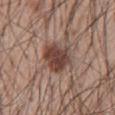<case>
<biopsy_status>not biopsied; imaged during a skin examination</biopsy_status>
<lighting>white-light</lighting>
<image>
  <source>total-body photography crop</source>
  <field_of_view_mm>15</field_of_view_mm>
</image>
<site>chest</site>
<patient>
  <sex>male</sex>
  <age_approx>55</age_approx>
</patient>
</case>